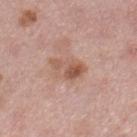workup: total-body-photography surveillance lesion; no biopsy
lighting: white-light
diameter: ~4 mm (longest diameter)
image source: ~15 mm crop, total-body skin-cancer survey
automated metrics: an area of roughly 8 mm² and an eccentricity of roughly 0.75; a lesion–skin lightness drop of about 9 and a lesion-to-skin contrast of about 7 (normalized; higher = more distinct); a border-irregularity index near 3/10, internal color variation of about 8.5 on a 0–10 scale, and a peripheral color-asymmetry measure near 3
anatomic site: the right lower leg
subject: female, aged around 50